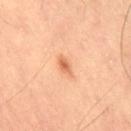Impression:
Imaged during a routine full-body skin examination; the lesion was not biopsied and no histopathology is available.
Context:
Cropped from a whole-body photographic skin survey; the tile spans about 15 mm. An algorithmic analysis of the crop reported a lesion area of about 3 mm² and a symmetry-axis asymmetry near 0.35. And it measured a lesion color around L≈68 a*≈28 b*≈41 in CIELAB and roughly 11 lightness units darker than nearby skin. The software also gave a border-irregularity rating of about 3/10 and internal color variation of about 2 on a 0–10 scale. The software also gave a nevus-likeness score of about 95/100 and a detector confidence of about 100 out of 100 that the crop contains a lesion. About 2.5 mm across. The patient is a male aged 53–57. From the right thigh. Captured under cross-polarized illumination.